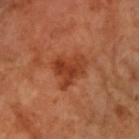notes: total-body-photography surveillance lesion; no biopsy
automated metrics: a footprint of about 11 mm², a shape eccentricity near 0.55, and a shape-asymmetry score of about 0.45 (0 = symmetric); a mean CIELAB color near L≈39 a*≈29 b*≈35, about 9 CIELAB-L* units darker than the surrounding skin, and a normalized border contrast of about 7.5; a within-lesion color-variation index near 4.5/10 and a peripheral color-asymmetry measure near 1.5; a nevus-likeness score of about 80/100 and a detector confidence of about 100 out of 100 that the crop contains a lesion
location: the arm
image: 15 mm crop, total-body photography
subject: female, approximately 70 years of age